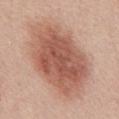– workup — imaged on a skin check; not biopsied
– anatomic site — the front of the torso
– tile lighting — white-light
– imaging modality — 15 mm crop, total-body photography
– patient — male, roughly 55 years of age
– TBP lesion metrics — a footprint of about 50 mm² and an eccentricity of roughly 0.8; a border-irregularity rating of about 2/10, internal color variation of about 5 on a 0–10 scale, and a peripheral color-asymmetry measure near 1.5; a classifier nevus-likeness of about 95/100 and lesion-presence confidence of about 100/100
– lesion size — about 10.5 mm